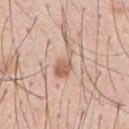Findings:
- notes: imaged on a skin check; not biopsied
- patient: male, aged 53 to 57
- lesion diameter: ≈4 mm
- imaging modality: ~15 mm tile from a whole-body skin photo
- tile lighting: white-light illumination
- automated lesion analysis: an area of roughly 5.5 mm², an outline eccentricity of about 0.9 (0 = round, 1 = elongated), and a shape-asymmetry score of about 0.6 (0 = symmetric); an average lesion color of about L≈61 a*≈20 b*≈30 (CIELAB), a lesion–skin lightness drop of about 11, and a normalized lesion–skin contrast near 7; a classifier nevus-likeness of about 80/100